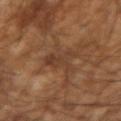Recorded during total-body skin imaging; not selected for excision or biopsy. Captured under cross-polarized illumination. The lesion-visualizer software estimated a footprint of about 7 mm² and a shape-asymmetry score of about 0.5 (0 = symmetric). The software also gave border irregularity of about 6.5 on a 0–10 scale and a within-lesion color-variation index near 2.5/10. It also reported a classifier nevus-likeness of about 0/100. The patient is a male approximately 55 years of age. Located on the right forearm. A close-up tile cropped from a whole-body skin photograph, about 15 mm across. The recorded lesion diameter is about 3.5 mm.